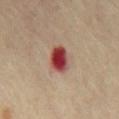Impression: The lesion was tiled from a total-body skin photograph and was not biopsied. Background: A close-up tile cropped from a whole-body skin photograph, about 15 mm across. The total-body-photography lesion software estimated a lesion area of about 7.5 mm², a shape eccentricity near 0.85, and a symmetry-axis asymmetry near 0.2. The software also gave a border-irregularity rating of about 2/10 and radial color variation of about 2. The software also gave a classifier nevus-likeness of about 0/100. A male patient, aged approximately 70. The lesion is on the chest. Imaged with cross-polarized lighting. Longest diameter approximately 4 mm.